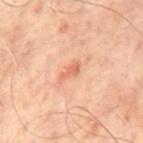Notes:
• follow-up: no biopsy performed (imaged during a skin exam)
• image-analysis metrics: a footprint of about 3 mm², a shape eccentricity near 0.9, and a shape-asymmetry score of about 0.45 (0 = symmetric); a mean CIELAB color near L≈64 a*≈27 b*≈35, roughly 9 lightness units darker than nearby skin, and a normalized lesion–skin contrast near 6; lesion-presence confidence of about 100/100
• illumination: cross-polarized
• subject: male, roughly 65 years of age
• location: the mid back
• imaging modality: 15 mm crop, total-body photography